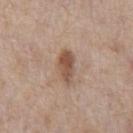Impression: Captured during whole-body skin photography for melanoma surveillance; the lesion was not biopsied. Background: A male patient, aged 63 to 67. The recorded lesion diameter is about 4 mm. This is a white-light tile. A region of skin cropped from a whole-body photographic capture, roughly 15 mm wide. From the chest.